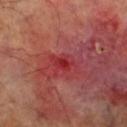{"lesion_size": {"long_diameter_mm_approx": 3.0}, "site": "leg", "lighting": "cross-polarized", "patient": {"sex": "male", "age_approx": 70}, "image": {"source": "total-body photography crop", "field_of_view_mm": 15}}